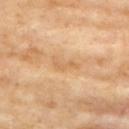Captured during whole-body skin photography for melanoma surveillance; the lesion was not biopsied. Captured under cross-polarized illumination. About 3.5 mm across. A close-up tile cropped from a whole-body skin photograph, about 15 mm across. A female patient, aged 73 to 77. Located on the chest.